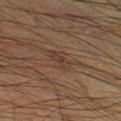Clinical impression: Captured during whole-body skin photography for melanoma surveillance; the lesion was not biopsied. Image and clinical context: The subject is a male aged around 35. This image is a 15 mm lesion crop taken from a total-body photograph. The tile uses cross-polarized illumination. The recorded lesion diameter is about 3.5 mm. From the left thigh.